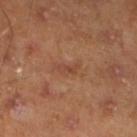Clinical impression:
The lesion was tiled from a total-body skin photograph and was not biopsied.
Context:
The lesion is on the left lower leg. A male patient, approximately 45 years of age. Measured at roughly 3 mm in maximum diameter. Cropped from a whole-body photographic skin survey; the tile spans about 15 mm.